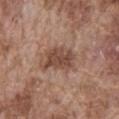Case summary:
– biopsy status · imaged on a skin check; not biopsied
– image source · 15 mm crop, total-body photography
– body site · the abdomen
– diameter · about 4.5 mm
– lighting · white-light illumination
– subject · male, approximately 75 years of age